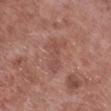Assessment: No biopsy was performed on this lesion — it was imaged during a full skin examination and was not determined to be concerning. Acquisition and patient details: Cropped from a total-body skin-imaging series; the visible field is about 15 mm. This is a white-light tile. A male patient aged approximately 55. The lesion is on the right lower leg.